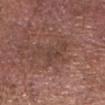– notes: total-body-photography surveillance lesion; no biopsy
– imaging modality: 15 mm crop, total-body photography
– site: the head or neck
– patient: male, aged around 75
– TBP lesion metrics: a lesion color around L≈42 a*≈18 b*≈24 in CIELAB, about 6 CIELAB-L* units darker than the surrounding skin, and a lesion-to-skin contrast of about 5.5 (normalized; higher = more distinct); a border-irregularity rating of about 9/10 and a color-variation rating of about 2/10; a lesion-detection confidence of about 75/100
– lesion size: ~5.5 mm (longest diameter)
– illumination: white-light illumination